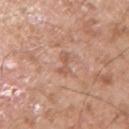notes: catalogued during a skin exam; not biopsied | diameter: ≈2.5 mm | anatomic site: the right upper arm | TBP lesion metrics: an eccentricity of roughly 0.95 and a shape-asymmetry score of about 0.4 (0 = symmetric); a border-irregularity index near 6/10, a within-lesion color-variation index near 0/10, and peripheral color asymmetry of about 0; a nevus-likeness score of about 0/100 and a lesion-detection confidence of about 100/100 | subject: male, aged around 55 | imaging modality: ~15 mm tile from a whole-body skin photo | illumination: white-light illumination.